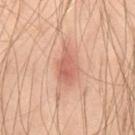Findings:
• biopsy status · total-body-photography surveillance lesion; no biopsy
• lighting · cross-polarized
• patient · male, roughly 45 years of age
• lesion size · about 4.5 mm
• image · ~15 mm tile from a whole-body skin photo
• automated lesion analysis · an area of roughly 7.5 mm², a shape eccentricity near 0.8, and a symmetry-axis asymmetry near 0.3; a mean CIELAB color near L≈60 a*≈26 b*≈30, a lesion–skin lightness drop of about 10, and a lesion-to-skin contrast of about 6.5 (normalized; higher = more distinct); a detector confidence of about 100 out of 100 that the crop contains a lesion
• location · the leg